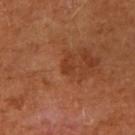Part of a total-body skin-imaging series; this lesion was reviewed on a skin check and was not flagged for biopsy. A 15 mm close-up extracted from a 3D total-body photography capture. A female subject, in their mid- to late 60s. On the right upper arm.